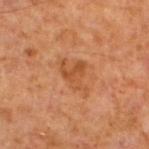Q: Is there a histopathology result?
A: no biopsy performed (imaged during a skin exam)